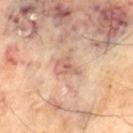Recorded during total-body skin imaging; not selected for excision or biopsy.